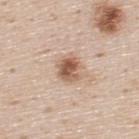Part of a total-body skin-imaging series; this lesion was reviewed on a skin check and was not flagged for biopsy. Longest diameter approximately 3 mm. The lesion is located on the upper back. A male subject in their mid- to late 40s. A region of skin cropped from a whole-body photographic capture, roughly 15 mm wide.